A close-up tile cropped from a whole-body skin photograph, about 15 mm across. Captured under white-light illumination. Located on the right lower leg. A female patient, in their mid-20s — 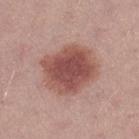* diagnosis · a dysplastic (Clark) nevus